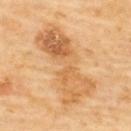workup=no biopsy performed (imaged during a skin exam)
subject=female, aged approximately 60
lesion size=~9.5 mm (longest diameter)
anatomic site=the upper back
image source=~15 mm tile from a whole-body skin photo
lighting=cross-polarized illumination